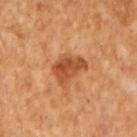| feature | finding |
|---|---|
| follow-up | total-body-photography surveillance lesion; no biopsy |
| acquisition | ~15 mm tile from a whole-body skin photo |
| automated lesion analysis | a shape eccentricity near 0.65 and two-axis asymmetry of about 0.35; a border-irregularity rating of about 3.5/10 and a peripheral color-asymmetry measure near 1.5 |
| lesion size | ~4 mm (longest diameter) |
| subject | male, in their mid-60s |
| tile lighting | cross-polarized illumination |